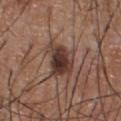| feature | finding |
|---|---|
| lesion size | about 5.5 mm |
| site | the front of the torso |
| subject | male, about 55 years old |
| acquisition | ~15 mm crop, total-body skin-cancer survey |
| lighting | white-light illumination |